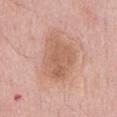Case summary:
• lesion size · ~6.5 mm (longest diameter)
• TBP lesion metrics · a footprint of about 19 mm², a shape eccentricity near 0.8, and a symmetry-axis asymmetry near 0.25; an average lesion color of about L≈61 a*≈21 b*≈30 (CIELAB), a lesion–skin lightness drop of about 9, and a lesion-to-skin contrast of about 6.5 (normalized; higher = more distinct); a color-variation rating of about 3.5/10 and peripheral color asymmetry of about 1.5; a classifier nevus-likeness of about 0/100 and a detector confidence of about 100 out of 100 that the crop contains a lesion
• anatomic site · the chest
• patient · male, aged around 60
• image source · total-body-photography crop, ~15 mm field of view